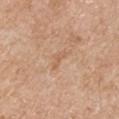The lesion was photographed on a routine skin check and not biopsied; there is no pathology result. Located on the right upper arm. The patient is a male aged around 55. Captured under white-light illumination. About 3 mm across. A 15 mm close-up extracted from a 3D total-body photography capture.